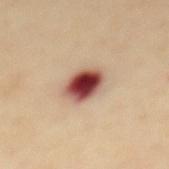Automated image analysis of the tile measured a footprint of about 9 mm².
On the mid back.
A roughly 15 mm field-of-view crop from a total-body skin photograph.
Measured at roughly 4 mm in maximum diameter.
Imaged with cross-polarized lighting.
The patient is a female aged approximately 40.